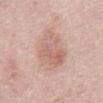Imaged during a routine full-body skin examination; the lesion was not biopsied and no histopathology is available. This image is a 15 mm lesion crop taken from a total-body photograph. Captured under white-light illumination. The recorded lesion diameter is about 6 mm. A male patient approximately 70 years of age. Located on the abdomen.